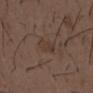The lesion was photographed on a routine skin check and not biopsied; there is no pathology result. A male patient approximately 50 years of age. Automated tile analysis of the lesion measured roughly 5 lightness units darker than nearby skin and a lesion-to-skin contrast of about 5 (normalized; higher = more distinct). It also reported a border-irregularity index near 2.5/10, a color-variation rating of about 2/10, and a peripheral color-asymmetry measure near 0.5. On the chest. A close-up tile cropped from a whole-body skin photograph, about 15 mm across. The recorded lesion diameter is about 3 mm. This is a white-light tile.